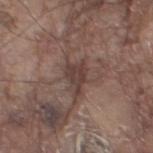Case summary:
* follow-up — total-body-photography surveillance lesion; no biopsy
* subject — male, about 80 years old
* automated lesion analysis — border irregularity of about 5 on a 0–10 scale and internal color variation of about 3 on a 0–10 scale
* image source — ~15 mm crop, total-body skin-cancer survey
* anatomic site — the left thigh